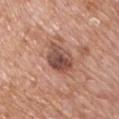Clinical impression: Imaged during a routine full-body skin examination; the lesion was not biopsied and no histopathology is available. Background: This is a white-light tile. A male patient aged 58–62. Cropped from a whole-body photographic skin survey; the tile spans about 15 mm. Located on the chest.